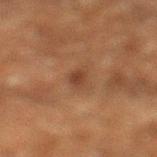Findings:
– follow-up · catalogued during a skin exam; not biopsied
– lighting · cross-polarized
– image · ~15 mm tile from a whole-body skin photo
– subject · male, roughly 60 years of age
– lesion diameter · about 2.5 mm
– TBP lesion metrics · a symmetry-axis asymmetry near 0.25; an automated nevus-likeness rating near 45 out of 100 and a detector confidence of about 100 out of 100 that the crop contains a lesion
– location · the left lower leg